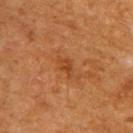Clinical impression: Recorded during total-body skin imaging; not selected for excision or biopsy. Context: The lesion is on the upper back. Cropped from a total-body skin-imaging series; the visible field is about 15 mm. The tile uses cross-polarized illumination. A male patient, aged 58 to 62. Longest diameter approximately 2.5 mm. Automated tile analysis of the lesion measured a mean CIELAB color near L≈41 a*≈25 b*≈37 and roughly 7 lightness units darker than nearby skin. The software also gave an automated nevus-likeness rating near 0 out of 100 and a lesion-detection confidence of about 100/100.